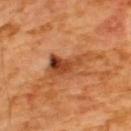Case summary:
– follow-up · catalogued during a skin exam; not biopsied
– patient · male, aged 63 to 67
– acquisition · ~15 mm crop, total-body skin-cancer survey
– tile lighting · cross-polarized
– location · the upper back